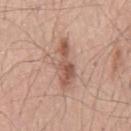<record>
  <biopsy_status>not biopsied; imaged during a skin examination</biopsy_status>
  <image>
    <source>total-body photography crop</source>
    <field_of_view_mm>15</field_of_view_mm>
  </image>
  <site>chest</site>
  <patient>
    <sex>male</sex>
    <age_approx>55</age_approx>
  </patient>
</record>